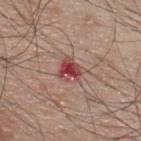notes — imaged on a skin check; not biopsied
site — the upper back
acquisition — total-body-photography crop, ~15 mm field of view
patient — male, aged 43–47
lighting — white-light illumination